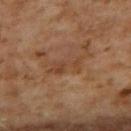A female patient, aged 58 to 62.
An algorithmic analysis of the crop reported a lesion area of about 5.5 mm². The software also gave a classifier nevus-likeness of about 0/100 and lesion-presence confidence of about 75/100.
The tile uses cross-polarized illumination.
A region of skin cropped from a whole-body photographic capture, roughly 15 mm wide.
From the upper back.